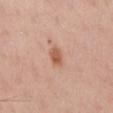Case summary:
- biopsy status — catalogued during a skin exam; not biopsied
- automated metrics — a border-irregularity index near 2.5/10, internal color variation of about 2.5 on a 0–10 scale, and peripheral color asymmetry of about 1
- lighting — white-light illumination
- image — ~15 mm crop, total-body skin-cancer survey
- anatomic site — the chest
- subject — male, in their 60s
- size — ≈2.5 mm